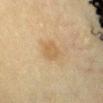{
  "biopsy_status": "not biopsied; imaged during a skin examination",
  "image": {
    "source": "total-body photography crop",
    "field_of_view_mm": 15
  },
  "site": "abdomen",
  "patient": {
    "sex": "female",
    "age_approx": 55
  }
}